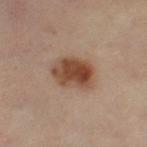{"biopsy_status": "not biopsied; imaged during a skin examination", "image": {"source": "total-body photography crop", "field_of_view_mm": 15}, "patient": {"sex": "female", "age_approx": 40}, "site": "left thigh"}